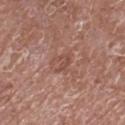| key | value |
|---|---|
| notes | catalogued during a skin exam; not biopsied |
| imaging modality | 15 mm crop, total-body photography |
| patient | male, aged 58–62 |
| tile lighting | white-light illumination |
| location | the left lower leg |
| automated lesion analysis | a border-irregularity rating of about 7.5/10, a within-lesion color-variation index near 0/10, and radial color variation of about 0; lesion-presence confidence of about 100/100 |
| size | about 2.5 mm |